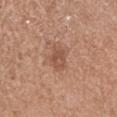follow-up = imaged on a skin check; not biopsied | lighting = white-light illumination | size = about 3 mm | subject = female, aged 68–72 | body site = the left forearm | image source = ~15 mm tile from a whole-body skin photo.